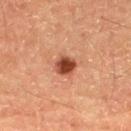<lesion>
  <biopsy_status>not biopsied; imaged during a skin examination</biopsy_status>
  <patient>
    <sex>male</sex>
    <age_approx>60</age_approx>
  </patient>
  <lighting>cross-polarized</lighting>
  <automated_metrics>
    <shape_asymmetry>0.15</shape_asymmetry>
    <border_irregularity_0_10>1.0</border_irregularity_0_10>
    <color_variation_0_10>3.5</color_variation_0_10>
    <peripheral_color_asymmetry>1.0</peripheral_color_asymmetry>
    <lesion_detection_confidence_0_100>100</lesion_detection_confidence_0_100>
  </automated_metrics>
  <lesion_size>
    <long_diameter_mm_approx>2.5</long_diameter_mm_approx>
  </lesion_size>
  <image>
    <source>total-body photography crop</source>
    <field_of_view_mm>15</field_of_view_mm>
  </image>
</lesion>